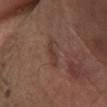Imaged during a routine full-body skin examination; the lesion was not biopsied and no histopathology is available. A male patient, aged 63 to 67. A roughly 15 mm field-of-view crop from a total-body skin photograph. Captured under cross-polarized illumination. From the left lower leg.